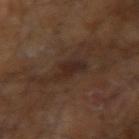{"biopsy_status": "not biopsied; imaged during a skin examination", "image": {"source": "total-body photography crop", "field_of_view_mm": 15}, "site": "right arm", "patient": {"sex": "male", "age_approx": 60}, "automated_metrics": {"area_mm2_approx": 7.0, "eccentricity": 0.85, "cielab_L": 24, "cielab_a": 15, "cielab_b": 20, "vs_skin_contrast_norm": 7.0, "color_variation_0_10": 4.0, "peripheral_color_asymmetry": 1.5, "nevus_likeness_0_100": 10, "lesion_detection_confidence_0_100": 95}, "lesion_size": {"long_diameter_mm_approx": 4.0}}